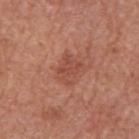Clinical impression:
This lesion was catalogued during total-body skin photography and was not selected for biopsy.
Context:
Imaged with white-light lighting. The subject is a female aged 63 to 67. The recorded lesion diameter is about 3.5 mm. Automated image analysis of the tile measured an area of roughly 6.5 mm². It also reported about 7 CIELAB-L* units darker than the surrounding skin and a normalized lesion–skin contrast near 5.5. The analysis additionally found border irregularity of about 4.5 on a 0–10 scale, a within-lesion color-variation index near 2/10, and radial color variation of about 1. Cropped from a whole-body photographic skin survey; the tile spans about 15 mm. The lesion is located on the left upper arm.